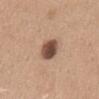The lesion was photographed on a routine skin check and not biopsied; there is no pathology result.
Imaged with white-light lighting.
A close-up tile cropped from a whole-body skin photograph, about 15 mm across.
Approximately 4 mm at its widest.
The patient is a male aged 33 to 37.
Located on the mid back.